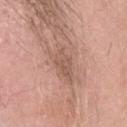biopsy_status: not biopsied; imaged during a skin examination
patient:
  sex: female
  age_approx: 60
lighting: white-light
image:
  source: total-body photography crop
  field_of_view_mm: 15
site: head or neck
lesion_size:
  long_diameter_mm_approx: 5.5